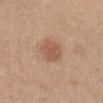Impression:
The lesion was photographed on a routine skin check and not biopsied; there is no pathology result.
Image and clinical context:
On the abdomen. Cropped from a total-body skin-imaging series; the visible field is about 15 mm. This is a white-light tile. The lesion's longest dimension is about 3.5 mm. The patient is a female aged 38 to 42.